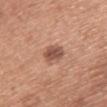  biopsy_status: not biopsied; imaged during a skin examination